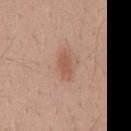No biopsy was performed on this lesion — it was imaged during a full skin examination and was not determined to be concerning. This image is a 15 mm lesion crop taken from a total-body photograph. A male patient aged approximately 40. Located on the back.